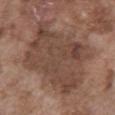biopsy status: catalogued during a skin exam; not biopsied